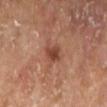lesion_size:
  long_diameter_mm_approx: 2.5
lighting: cross-polarized
automated_metrics:
  border_irregularity_0_10: 2.0
  color_variation_0_10: 2.5
  peripheral_color_asymmetry: 1.0
  nevus_likeness_0_100: 65
  lesion_detection_confidence_0_100: 100
image:
  source: total-body photography crop
  field_of_view_mm: 15
site: right lower leg
patient:
  sex: female
  age_approx: 75